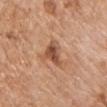workup — total-body-photography surveillance lesion; no biopsy | imaging modality — 15 mm crop, total-body photography | patient — male, approximately 60 years of age | anatomic site — the front of the torso | TBP lesion metrics — an average lesion color of about L≈51 a*≈23 b*≈32 (CIELAB), about 12 CIELAB-L* units darker than the surrounding skin, and a normalized border contrast of about 8.5; a border-irregularity index near 4/10, a color-variation rating of about 4.5/10, and radial color variation of about 1.5; a classifier nevus-likeness of about 65/100 and lesion-presence confidence of about 100/100.